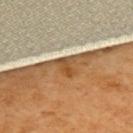{
  "biopsy_status": "not biopsied; imaged during a skin examination",
  "image": {
    "source": "total-body photography crop",
    "field_of_view_mm": 15
  },
  "lesion_size": {
    "long_diameter_mm_approx": 2.5
  },
  "lighting": "cross-polarized",
  "patient": {
    "sex": "female",
    "age_approx": 55
  },
  "site": "upper back",
  "automated_metrics": {
    "border_irregularity_0_10": 3.5,
    "color_variation_0_10": 4.0,
    "peripheral_color_asymmetry": 1.5,
    "nevus_likeness_0_100": 0,
    "lesion_detection_confidence_0_100": 75
  }
}